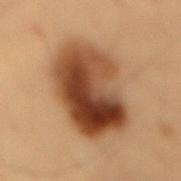– body site · the back
– acquisition · ~15 mm crop, total-body skin-cancer survey
– patient · male, about 55 years old
– tile lighting · cross-polarized illumination
– lesion size · about 8.5 mm